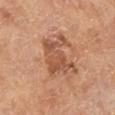The lesion was photographed on a routine skin check and not biopsied; there is no pathology result. The tile uses cross-polarized illumination. An algorithmic analysis of the crop reported a normalized border contrast of about 7. And it measured a nevus-likeness score of about 5/100 and a detector confidence of about 100 out of 100 that the crop contains a lesion. Located on the right lower leg. This image is a 15 mm lesion crop taken from a total-body photograph. A male patient about 65 years old.